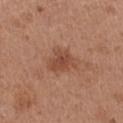notes — catalogued during a skin exam; not biopsied | size — ~3.5 mm (longest diameter) | automated lesion analysis — a border-irregularity rating of about 3/10, internal color variation of about 2.5 on a 0–10 scale, and a peripheral color-asymmetry measure near 0.5; an automated nevus-likeness rating near 40 out of 100 and a lesion-detection confidence of about 100/100 | patient — female, about 40 years old | anatomic site — the leg | image — 15 mm crop, total-body photography.